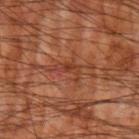The lesion was tiled from a total-body skin photograph and was not biopsied.
Approximately 3.5 mm at its widest.
Imaged with cross-polarized lighting.
The lesion is on the left upper arm.
The patient is a male roughly 60 years of age.
Automated tile analysis of the lesion measured a lesion area of about 4 mm², an eccentricity of roughly 0.85, and a symmetry-axis asymmetry near 0.6. The software also gave a lesion color around L≈39 a*≈26 b*≈32 in CIELAB. And it measured a nevus-likeness score of about 0/100.
A lesion tile, about 15 mm wide, cut from a 3D total-body photograph.